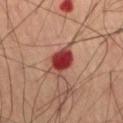anatomic site: the right thigh | automated metrics: a lesion area of about 8 mm², an outline eccentricity of about 0.75 (0 = round, 1 = elongated), and a shape-asymmetry score of about 0.2 (0 = symmetric); a border-irregularity index near 2.5/10, internal color variation of about 6.5 on a 0–10 scale, and peripheral color asymmetry of about 2 | imaging modality: ~15 mm tile from a whole-body skin photo | patient: male, aged 68–72 | lesion size: about 4 mm | lighting: cross-polarized illumination.